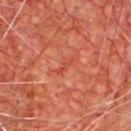notes = total-body-photography surveillance lesion; no biopsy
site = the chest
tile lighting = cross-polarized illumination
patient = male, in their mid-60s
acquisition = total-body-photography crop, ~15 mm field of view
image-analysis metrics = a border-irregularity index near 5/10, internal color variation of about 0 on a 0–10 scale, and radial color variation of about 0; a nevus-likeness score of about 0/100 and a lesion-detection confidence of about 95/100
diameter = ~2.5 mm (longest diameter)